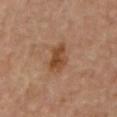<tbp_lesion>
<biopsy_status>not biopsied; imaged during a skin examination</biopsy_status>
<patient>
  <sex>male</sex>
  <age_approx>70</age_approx>
</patient>
<lighting>cross-polarized</lighting>
<site>mid back</site>
<automated_metrics>
  <area_mm2_approx>8.0</area_mm2_approx>
  <eccentricity>0.85</eccentricity>
  <border_irregularity_0_10>3.0</border_irregularity_0_10>
  <color_variation_0_10>4.5</color_variation_0_10>
  <lesion_detection_confidence_0_100>100</lesion_detection_confidence_0_100>
</automated_metrics>
<image>
  <source>total-body photography crop</source>
  <field_of_view_mm>15</field_of_view_mm>
</image>
</tbp_lesion>